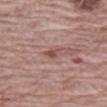{"biopsy_status": "not biopsied; imaged during a skin examination", "image": {"source": "total-body photography crop", "field_of_view_mm": 15}, "patient": {"sex": "male", "age_approx": 70}, "lighting": "white-light", "site": "left thigh"}